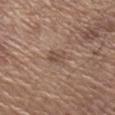{"biopsy_status": "not biopsied; imaged during a skin examination", "lighting": "white-light", "automated_metrics": {"area_mm2_approx": 4.5, "shape_asymmetry": 0.15, "vs_skin_darker_L": 7.0, "nevus_likeness_0_100": 0}, "patient": {"sex": "male", "age_approx": 65}, "image": {"source": "total-body photography crop", "field_of_view_mm": 15}, "site": "arm"}